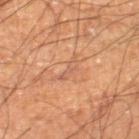biopsy status = no biopsy performed (imaged during a skin exam).